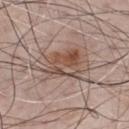Clinical impression:
Imaged during a routine full-body skin examination; the lesion was not biopsied and no histopathology is available.
Image and clinical context:
A 15 mm close-up tile from a total-body photography series done for melanoma screening. On the chest. Captured under white-light illumination. About 7 mm across. A male patient, aged 63–67. An algorithmic analysis of the crop reported a footprint of about 23 mm², an outline eccentricity of about 0.8 (0 = round, 1 = elongated), and two-axis asymmetry of about 0.25. It also reported about 10 CIELAB-L* units darker than the surrounding skin and a normalized lesion–skin contrast near 7. The software also gave border irregularity of about 4.5 on a 0–10 scale, internal color variation of about 8 on a 0–10 scale, and radial color variation of about 2.5.